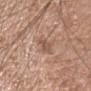Clinical impression:
This lesion was catalogued during total-body skin photography and was not selected for biopsy.
Background:
A lesion tile, about 15 mm wide, cut from a 3D total-body photograph. The lesion is on the upper back. Approximately 3 mm at its widest. A female patient in their 70s. This is a white-light tile.